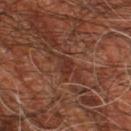No biopsy was performed on this lesion — it was imaged during a full skin examination and was not determined to be concerning. A roughly 15 mm field-of-view crop from a total-body skin photograph. A male subject approximately 60 years of age. The lesion is on the right leg.